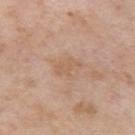workup: catalogued during a skin exam; not biopsied.